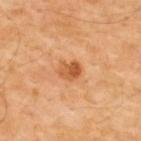follow-up=total-body-photography surveillance lesion; no biopsy
image-analysis metrics=an area of roughly 5 mm², an eccentricity of roughly 0.6, and a symmetry-axis asymmetry near 0.2; a within-lesion color-variation index near 5.5/10 and a peripheral color-asymmetry measure near 2
imaging modality=~15 mm tile from a whole-body skin photo
anatomic site=the upper back
subject=male, in their mid-50s
lesion diameter=~2.5 mm (longest diameter)
tile lighting=cross-polarized illumination